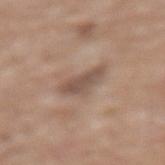biopsy status: catalogued during a skin exam; not biopsied | lesion diameter: ~4 mm (longest diameter) | lighting: white-light | patient: male, aged 78–82 | imaging modality: total-body-photography crop, ~15 mm field of view | anatomic site: the lower back.